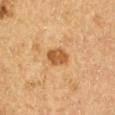notes: no biopsy performed (imaged during a skin exam) | diameter: ≈3 mm | patient: male, in their mid-60s | illumination: cross-polarized | imaging modality: ~15 mm crop, total-body skin-cancer survey | anatomic site: the right upper arm | TBP lesion metrics: an area of roughly 5.5 mm², a shape eccentricity near 0.75, and a shape-asymmetry score of about 0.2 (0 = symmetric); a nevus-likeness score of about 75/100.